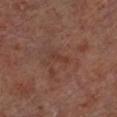Impression:
Part of a total-body skin-imaging series; this lesion was reviewed on a skin check and was not flagged for biopsy.
Background:
Measured at roughly 2.5 mm in maximum diameter. The tile uses cross-polarized illumination. From the left lower leg. A roughly 15 mm field-of-view crop from a total-body skin photograph. Automated tile analysis of the lesion measured a footprint of about 2 mm², a shape eccentricity near 0.95, and two-axis asymmetry of about 0.35. The software also gave an average lesion color of about L≈35 a*≈21 b*≈25 (CIELAB), about 5 CIELAB-L* units darker than the surrounding skin, and a normalized lesion–skin contrast near 5. The software also gave a border-irregularity rating of about 4/10, a within-lesion color-variation index near 0/10, and radial color variation of about 0. A male subject aged approximately 65.